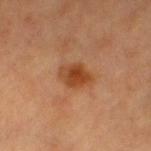notes: no biopsy performed (imaged during a skin exam)
patient: female, aged approximately 40
image source: ~15 mm tile from a whole-body skin photo
body site: the left lower leg